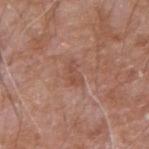Case summary:
– follow-up · total-body-photography surveillance lesion; no biopsy
– patient · male, aged 58–62
– TBP lesion metrics · a nevus-likeness score of about 0/100
– image · ~15 mm crop, total-body skin-cancer survey
– lesion diameter · ≈2.5 mm
– tile lighting · white-light illumination
– body site · the right upper arm